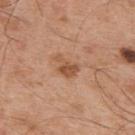acquisition = ~15 mm tile from a whole-body skin photo
location = the upper back
lesion size = ≈3 mm
subject = male, aged 53–57